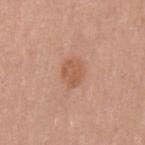follow-up: total-body-photography surveillance lesion; no biopsy | diameter: ≈3 mm | tile lighting: white-light illumination | image source: ~15 mm crop, total-body skin-cancer survey | body site: the mid back | image-analysis metrics: a lesion area of about 5 mm², a shape eccentricity near 0.7, and a symmetry-axis asymmetry near 0.25; an average lesion color of about L≈56 a*≈23 b*≈32 (CIELAB) and about 8 CIELAB-L* units darker than the surrounding skin; a classifier nevus-likeness of about 30/100 and lesion-presence confidence of about 100/100 | patient: male, approximately 45 years of age.